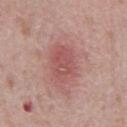workup: no biopsy performed (imaged during a skin exam); image-analysis metrics: an eccentricity of roughly 0.8 and a shape-asymmetry score of about 0.2 (0 = symmetric); subject: male, approximately 70 years of age; image: 15 mm crop, total-body photography; anatomic site: the abdomen; lighting: white-light.